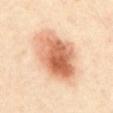Impression:
Recorded during total-body skin imaging; not selected for excision or biopsy.
Background:
A male subject roughly 40 years of age. The lesion is on the abdomen. A lesion tile, about 15 mm wide, cut from a 3D total-body photograph.